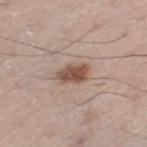notes: imaged on a skin check; not biopsied | location: the right leg | image: total-body-photography crop, ~15 mm field of view | subject: male, about 70 years old | TBP lesion metrics: a border-irregularity rating of about 2.5/10 and a within-lesion color-variation index near 3.5/10; an automated nevus-likeness rating near 95 out of 100 and a lesion-detection confidence of about 100/100.